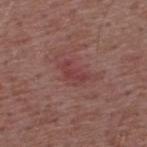Assessment: Part of a total-body skin-imaging series; this lesion was reviewed on a skin check and was not flagged for biopsy. Background: This is a white-light tile. A roughly 15 mm field-of-view crop from a total-body skin photograph. A male patient roughly 55 years of age. Located on the upper back.